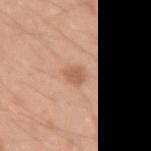notes — catalogued during a skin exam; not biopsied | lesion diameter — about 2.5 mm | location — the left upper arm | automated metrics — a lesion area of about 3.5 mm², an eccentricity of roughly 0.75, and a symmetry-axis asymmetry near 0.3; a lesion–skin lightness drop of about 9 and a lesion-to-skin contrast of about 6 (normalized; higher = more distinct) | illumination — white-light illumination | patient — male, aged around 20 | acquisition — ~15 mm tile from a whole-body skin photo.